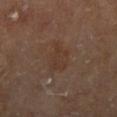Assessment: The lesion was tiled from a total-body skin photograph and was not biopsied. Context: A female patient, aged around 75. On the left lower leg. A 15 mm crop from a total-body photograph taken for skin-cancer surveillance. The lesion's longest dimension is about 4 mm. The tile uses cross-polarized illumination.